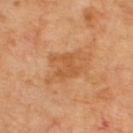biopsy status = catalogued during a skin exam; not biopsied
image = 15 mm crop, total-body photography
patient = approximately 65 years of age
body site = the upper back